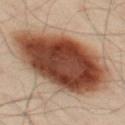Clinical impression: Recorded during total-body skin imaging; not selected for excision or biopsy. Acquisition and patient details: On the left thigh. The lesion's longest dimension is about 12.5 mm. A lesion tile, about 15 mm wide, cut from a 3D total-body photograph. A male subject, roughly 50 years of age. Captured under cross-polarized illumination.